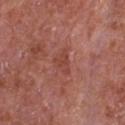Q: Was this lesion biopsied?
A: no biopsy performed (imaged during a skin exam)
Q: What are the patient's age and sex?
A: male, aged approximately 65
Q: What kind of image is this?
A: total-body-photography crop, ~15 mm field of view
Q: Where on the body is the lesion?
A: the chest
Q: How large is the lesion?
A: about 3 mm